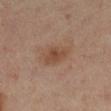follow-up: no biopsy performed (imaged during a skin exam)
subject: female, aged approximately 40
tile lighting: cross-polarized illumination
site: the left leg
image-analysis metrics: an area of roughly 6 mm² and an outline eccentricity of about 0.6 (0 = round, 1 = elongated); a classifier nevus-likeness of about 75/100 and a lesion-detection confidence of about 100/100
image source: ~15 mm crop, total-body skin-cancer survey